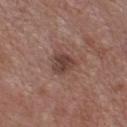Findings:
– biopsy status: imaged on a skin check; not biopsied
– patient: male, aged around 55
– location: the chest
– illumination: white-light illumination
– imaging modality: total-body-photography crop, ~15 mm field of view